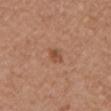Findings:
– lighting — white-light illumination
– lesion diameter — about 2.5 mm
– imaging modality — ~15 mm tile from a whole-body skin photo
– subject — female, approximately 70 years of age
– location — the right upper arm